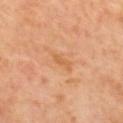Imaged during a routine full-body skin examination; the lesion was not biopsied and no histopathology is available. The lesion is on the upper back. A 15 mm crop from a total-body photograph taken for skin-cancer surveillance. The recorded lesion diameter is about 2.5 mm.